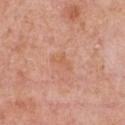workup: imaged on a skin check; not biopsied
tile lighting: white-light
body site: the chest
automated metrics: a footprint of about 3.5 mm², an outline eccentricity of about 0.8 (0 = round, 1 = elongated), and two-axis asymmetry of about 0.5; a border-irregularity index near 5/10, internal color variation of about 1.5 on a 0–10 scale, and peripheral color asymmetry of about 0.5
patient: female, aged 38–42
image: ~15 mm tile from a whole-body skin photo